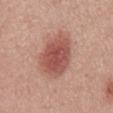Assessment: Part of a total-body skin-imaging series; this lesion was reviewed on a skin check and was not flagged for biopsy. Background: Located on the mid back. A male subject, aged 28 to 32. A 15 mm close-up tile from a total-body photography series done for melanoma screening.